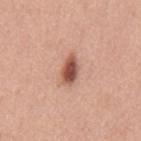Q: Is there a histopathology result?
A: total-body-photography surveillance lesion; no biopsy
Q: Lesion location?
A: the mid back
Q: How was this image acquired?
A: ~15 mm crop, total-body skin-cancer survey
Q: What lighting was used for the tile?
A: white-light illumination
Q: How large is the lesion?
A: ≈3.5 mm
Q: Who is the patient?
A: male, in their 60s